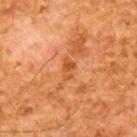The lesion was photographed on a routine skin check and not biopsied; there is no pathology result. Imaged with cross-polarized lighting. A male patient, in their mid-60s. A 15 mm close-up extracted from a 3D total-body photography capture. About 2.5 mm across.